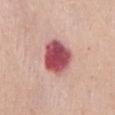{
  "lighting": "white-light",
  "image": {
    "source": "total-body photography crop",
    "field_of_view_mm": 15
  },
  "lesion_size": {
    "long_diameter_mm_approx": 4.5
  },
  "site": "abdomen",
  "patient": {
    "sex": "female",
    "age_approx": 70
  }
}